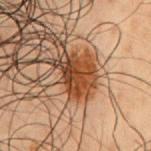Q: Was this lesion biopsied?
A: catalogued during a skin exam; not biopsied
Q: What is the anatomic site?
A: the front of the torso
Q: What are the patient's age and sex?
A: male, about 50 years old
Q: What is the imaging modality?
A: total-body-photography crop, ~15 mm field of view